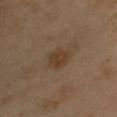Part of a total-body skin-imaging series; this lesion was reviewed on a skin check and was not flagged for biopsy.
A close-up tile cropped from a whole-body skin photograph, about 15 mm across.
A female subject, approximately 40 years of age.
On the chest.